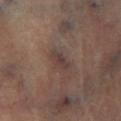The lesion was photographed on a routine skin check and not biopsied; there is no pathology result.
A male subject aged around 65.
This is a cross-polarized tile.
The lesion is located on the right lower leg.
The lesion's longest dimension is about 3 mm.
The total-body-photography lesion software estimated a border-irregularity rating of about 2.5/10, internal color variation of about 3 on a 0–10 scale, and radial color variation of about 1.
A 15 mm close-up tile from a total-body photography series done for melanoma screening.